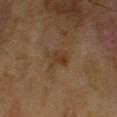{
  "biopsy_status": "not biopsied; imaged during a skin examination",
  "lighting": "cross-polarized",
  "patient": {
    "sex": "male",
    "age_approx": 65
  },
  "lesion_size": {
    "long_diameter_mm_approx": 3.0
  },
  "automated_metrics": {
    "eccentricity": 0.65,
    "shape_asymmetry": 0.25,
    "vs_skin_darker_L": 6.0
  },
  "image": {
    "source": "total-body photography crop",
    "field_of_view_mm": 15
  }
}